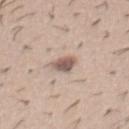Recorded during total-body skin imaging; not selected for excision or biopsy.
Cropped from a whole-body photographic skin survey; the tile spans about 15 mm.
The patient is a male in their 40s.
About 2.5 mm across.
An algorithmic analysis of the crop reported a lesion area of about 4.5 mm², an eccentricity of roughly 0.5, and two-axis asymmetry of about 0.25. The analysis additionally found a classifier nevus-likeness of about 80/100 and a lesion-detection confidence of about 100/100.
Imaged with white-light lighting.
The lesion is located on the abdomen.